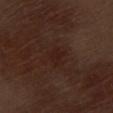Q: Was a biopsy performed?
A: total-body-photography surveillance lesion; no biopsy
Q: Automated lesion metrics?
A: a footprint of about 5 mm² and an outline eccentricity of about 0.65 (0 = round, 1 = elongated); border irregularity of about 3 on a 0–10 scale, internal color variation of about 1.5 on a 0–10 scale, and peripheral color asymmetry of about 0.5
Q: How large is the lesion?
A: ~3 mm (longest diameter)
Q: What are the patient's age and sex?
A: male, about 70 years old
Q: What kind of image is this?
A: ~15 mm tile from a whole-body skin photo
Q: Where on the body is the lesion?
A: the left thigh
Q: Illumination type?
A: white-light